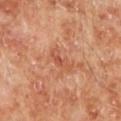| key | value |
|---|---|
| follow-up | no biopsy performed (imaged during a skin exam) |
| subject | male, aged 68 to 72 |
| illumination | cross-polarized |
| lesion size | about 3 mm |
| image | total-body-photography crop, ~15 mm field of view |
| location | the leg |